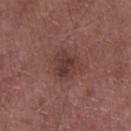* follow-up · imaged on a skin check; not biopsied
* image source · 15 mm crop, total-body photography
* lighting · white-light illumination
* patient · male, aged around 55
* location · the left lower leg
* TBP lesion metrics · a mean CIELAB color near L≈37 a*≈21 b*≈21 and roughly 8 lightness units darker than nearby skin; a border-irregularity index near 2/10, a within-lesion color-variation index near 3.5/10, and a peripheral color-asymmetry measure near 1.5
* size · ≈3.5 mm